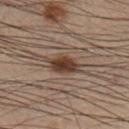Impression:
The lesion was photographed on a routine skin check and not biopsied; there is no pathology result.
Image and clinical context:
The lesion-visualizer software estimated a lesion area of about 7 mm², an outline eccentricity of about 0.75 (0 = round, 1 = elongated), and a symmetry-axis asymmetry near 0.15. The software also gave a lesion–skin lightness drop of about 10 and a lesion-to-skin contrast of about 10.5 (normalized; higher = more distinct). The analysis additionally found a border-irregularity rating of about 2/10, internal color variation of about 3 on a 0–10 scale, and a peripheral color-asymmetry measure near 1. A 15 mm close-up extracted from a 3D total-body photography capture. The lesion is located on the right lower leg. Longest diameter approximately 4 mm. The tile uses cross-polarized illumination. A male patient in their mid- to late 50s.